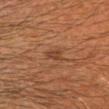Clinical impression:
Part of a total-body skin-imaging series; this lesion was reviewed on a skin check and was not flagged for biopsy.
Acquisition and patient details:
A 15 mm close-up extracted from a 3D total-body photography capture. A male subject roughly 60 years of age. The tile uses cross-polarized illumination. The lesion is on the head or neck. The lesion-visualizer software estimated a within-lesion color-variation index near 2.5/10. It also reported a nevus-likeness score of about 15/100.